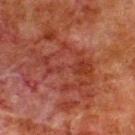Captured during whole-body skin photography for melanoma surveillance; the lesion was not biopsied. Imaged with cross-polarized lighting. A 15 mm crop from a total-body photograph taken for skin-cancer surveillance. Located on the upper back. Longest diameter approximately 8.5 mm. A male patient, about 80 years old.